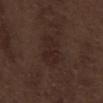Findings:
- biopsy status — total-body-photography surveillance lesion; no biopsy
- automated metrics — a footprint of about 10 mm²
- patient — male, about 70 years old
- lighting — white-light illumination
- lesion size — ≈5 mm
- location — the abdomen
- image source — total-body-photography crop, ~15 mm field of view